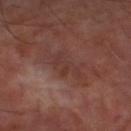This lesion was catalogued during total-body skin photography and was not selected for biopsy.
An algorithmic analysis of the crop reported an area of roughly 6.5 mm², a shape eccentricity near 0.85, and two-axis asymmetry of about 0.45. The analysis additionally found a lesion–skin lightness drop of about 5. The analysis additionally found an automated nevus-likeness rating near 0 out of 100 and lesion-presence confidence of about 90/100.
Imaged with cross-polarized lighting.
A 15 mm crop from a total-body photograph taken for skin-cancer surveillance.
Located on the left thigh.
Approximately 4 mm at its widest.
A male patient, in their 70s.